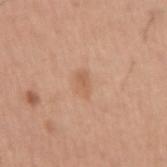Recorded during total-body skin imaging; not selected for excision or biopsy. The lesion's longest dimension is about 3 mm. A male subject aged approximately 60. A 15 mm crop from a total-body photograph taken for skin-cancer surveillance. The lesion is located on the right upper arm. Automated image analysis of the tile measured a lesion area of about 3.5 mm², an eccentricity of roughly 0.85, and a shape-asymmetry score of about 0.35 (0 = symmetric). It also reported a lesion–skin lightness drop of about 7 and a normalized border contrast of about 5. The software also gave a border-irregularity rating of about 3/10, internal color variation of about 1 on a 0–10 scale, and a peripheral color-asymmetry measure near 0.5.